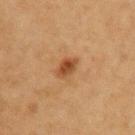  automated_metrics:
    cielab_L: 41
    cielab_a: 21
    cielab_b: 34
    vs_skin_darker_L: 11.0
    border_irregularity_0_10: 2.0
    color_variation_0_10: 3.0
    peripheral_color_asymmetry: 1.0
    nevus_likeness_0_100: 90
    lesion_detection_confidence_0_100: 100
  site: left upper arm
  image:
    source: total-body photography crop
    field_of_view_mm: 15
  lighting: cross-polarized
  lesion_size:
    long_diameter_mm_approx: 3.0
  patient:
    sex: female
    age_approx: 40